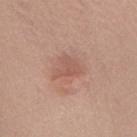workup: catalogued during a skin exam; not biopsied
illumination: white-light illumination
image source: total-body-photography crop, ~15 mm field of view
image-analysis metrics: a shape-asymmetry score of about 0.5 (0 = symmetric); a lesion color around L≈55 a*≈22 b*≈27 in CIELAB; a border-irregularity index near 5/10, a within-lesion color-variation index near 2/10, and radial color variation of about 0.5; a classifier nevus-likeness of about 40/100 and a lesion-detection confidence of about 100/100
patient: female, aged approximately 65
location: the upper back